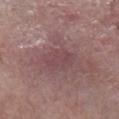biopsy status=imaged on a skin check; not biopsied
lesion diameter=≈4 mm
subject=male, aged around 80
site=the left lower leg
acquisition=15 mm crop, total-body photography
lighting=white-light